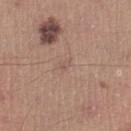workup: imaged on a skin check; not biopsied
patient: male, in their mid- to late 40s
acquisition: ~15 mm crop, total-body skin-cancer survey
lighting: white-light illumination
size: ~1.5 mm (longest diameter)
body site: the left lower leg
TBP lesion metrics: a lesion area of about 1.5 mm², an outline eccentricity of about 0.75 (0 = round, 1 = elongated), and a shape-asymmetry score of about 0.4 (0 = symmetric); an automated nevus-likeness rating near 0 out of 100 and a detector confidence of about 100 out of 100 that the crop contains a lesion